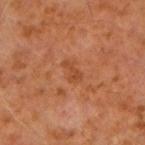The lesion was tiled from a total-body skin photograph and was not biopsied.
A male patient, aged around 60.
About 2.5 mm across.
A roughly 15 mm field-of-view crop from a total-body skin photograph.
The lesion is on the right forearm.